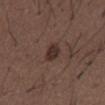biopsy status: no biopsy performed (imaged during a skin exam) | acquisition: ~15 mm tile from a whole-body skin photo | lesion diameter: about 3 mm | TBP lesion metrics: an area of roughly 5 mm² and a shape-asymmetry score of about 0.15 (0 = symmetric); a lesion color around L≈31 a*≈16 b*≈20 in CIELAB, a lesion–skin lightness drop of about 9, and a normalized border contrast of about 9; a color-variation rating of about 2.5/10 and a peripheral color-asymmetry measure near 1; lesion-presence confidence of about 100/100 | subject: male, aged approximately 50 | body site: the back.